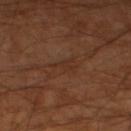The lesion was tiled from a total-body skin photograph and was not biopsied.
This is a cross-polarized tile.
Automated image analysis of the tile measured a footprint of about 2.5 mm², an eccentricity of roughly 0.75, and a symmetry-axis asymmetry near 0.6. And it measured a mean CIELAB color near L≈29 a*≈19 b*≈26, roughly 5 lightness units darker than nearby skin, and a normalized lesion–skin contrast near 5. The software also gave a border-irregularity index near 6.5/10 and a within-lesion color-variation index near 0/10.
The subject is a male aged 58–62.
A region of skin cropped from a whole-body photographic capture, roughly 15 mm wide.
Longest diameter approximately 2.5 mm.
On the left lower leg.